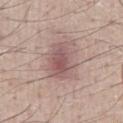biopsy status: catalogued during a skin exam; not biopsied
acquisition: 15 mm crop, total-body photography
site: the front of the torso
patient: male, approximately 50 years of age